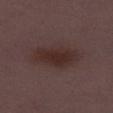No biopsy was performed on this lesion — it was imaged during a full skin examination and was not determined to be concerning. The lesion is located on the right thigh. A 15 mm crop from a total-body photograph taken for skin-cancer surveillance. The recorded lesion diameter is about 6 mm. The subject is a female aged 28 to 32.